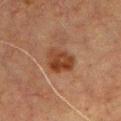follow-up: no biopsy performed (imaged during a skin exam)
imaging modality: 15 mm crop, total-body photography
automated metrics: a lesion area of about 8.5 mm², a shape eccentricity near 0.6, and a shape-asymmetry score of about 0.25 (0 = symmetric); a mean CIELAB color near L≈32 a*≈20 b*≈28, roughly 9 lightness units darker than nearby skin, and a normalized lesion–skin contrast near 9; a nevus-likeness score of about 85/100 and a lesion-detection confidence of about 100/100
anatomic site: the chest
subject: male, aged 48 to 52
lighting: cross-polarized illumination